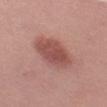site = the left thigh; subject = female, in their 40s; image = ~15 mm tile from a whole-body skin photo.